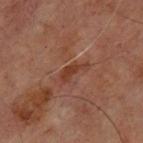Captured during whole-body skin photography for melanoma surveillance; the lesion was not biopsied. A region of skin cropped from a whole-body photographic capture, roughly 15 mm wide. The lesion is located on the chest. The subject is a male aged 68 to 72.